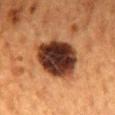follow-up: catalogued during a skin exam; not biopsied | diameter: about 6 mm | image source: ~15 mm tile from a whole-body skin photo | subject: female, in their 50s | illumination: cross-polarized | location: the mid back.